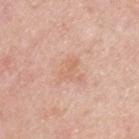Imaged during a routine full-body skin examination; the lesion was not biopsied and no histopathology is available. An algorithmic analysis of the crop reported a lesion area of about 3 mm² and a symmetry-axis asymmetry near 0.4. It also reported peripheral color asymmetry of about 0. It also reported a nevus-likeness score of about 0/100 and a detector confidence of about 100 out of 100 that the crop contains a lesion. From the upper back. Cropped from a whole-body photographic skin survey; the tile spans about 15 mm. The lesion's longest dimension is about 2.5 mm. A male patient aged around 45.